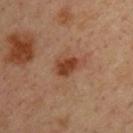| feature | finding |
|---|---|
| follow-up | no biopsy performed (imaged during a skin exam) |
| anatomic site | the upper back |
| subject | male, approximately 35 years of age |
| illumination | cross-polarized illumination |
| image | total-body-photography crop, ~15 mm field of view |